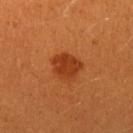| feature | finding |
|---|---|
| biopsy status | no biopsy performed (imaged during a skin exam) |
| lighting | cross-polarized illumination |
| patient | female, aged 28–32 |
| image source | ~15 mm tile from a whole-body skin photo |
| automated metrics | a lesion area of about 7.5 mm² and a shape-asymmetry score of about 0.2 (0 = symmetric); a lesion color around L≈36 a*≈30 b*≈39 in CIELAB, roughly 10 lightness units darker than nearby skin, and a normalized border contrast of about 8; lesion-presence confidence of about 100/100 |
| body site | the left upper arm |